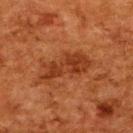{
  "biopsy_status": "not biopsied; imaged during a skin examination",
  "automated_metrics": {
    "area_mm2_approx": 12.0,
    "eccentricity": 0.9,
    "shape_asymmetry": 0.3
  },
  "site": "upper back",
  "image": {
    "source": "total-body photography crop",
    "field_of_view_mm": 15
  },
  "patient": {
    "sex": "female",
    "age_approx": 50
  }
}